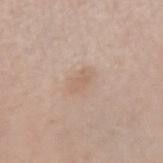This lesion was catalogued during total-body skin photography and was not selected for biopsy.